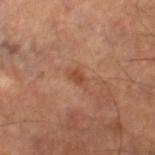Impression:
The lesion was tiled from a total-body skin photograph and was not biopsied.
Context:
The tile uses cross-polarized illumination. The subject is a male aged around 60. About 2 mm across. A 15 mm close-up extracted from a 3D total-body photography capture. The lesion is located on the right lower leg. The total-body-photography lesion software estimated an area of roughly 2 mm² and two-axis asymmetry of about 0.35. The software also gave an average lesion color of about L≈44 a*≈24 b*≈32 (CIELAB), about 8 CIELAB-L* units darker than the surrounding skin, and a normalized lesion–skin contrast near 7. It also reported a border-irregularity rating of about 3/10, internal color variation of about 0 on a 0–10 scale, and peripheral color asymmetry of about 0.